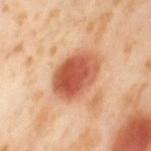Q: Is there a histopathology result?
A: total-body-photography surveillance lesion; no biopsy
Q: Lesion location?
A: the leg
Q: How was the tile lit?
A: cross-polarized
Q: What are the patient's age and sex?
A: female, in their mid- to late 50s
Q: How large is the lesion?
A: ≈5.5 mm
Q: What is the imaging modality?
A: ~15 mm crop, total-body skin-cancer survey